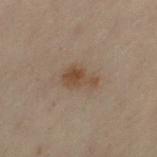Recorded during total-body skin imaging; not selected for excision or biopsy.
A 15 mm crop from a total-body photograph taken for skin-cancer surveillance.
Automated image analysis of the tile measured an outline eccentricity of about 0.75 (0 = round, 1 = elongated) and a symmetry-axis asymmetry near 0.3. And it measured an automated nevus-likeness rating near 80 out of 100 and lesion-presence confidence of about 100/100.
On the left thigh.
The patient is a female aged around 50.